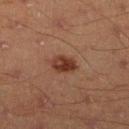  biopsy_status: not biopsied; imaged during a skin examination
  patient:
    sex: male
    age_approx: 45
  image:
    source: total-body photography crop
    field_of_view_mm: 15
  lighting: cross-polarized
  lesion_size:
    long_diameter_mm_approx: 3.0
  site: right lower leg
  automated_metrics:
    area_mm2_approx: 6.0
    eccentricity: 0.65
    shape_asymmetry: 0.25
    border_irregularity_0_10: 2.0
    color_variation_0_10: 4.5